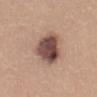The lesion was photographed on a routine skin check and not biopsied; there is no pathology result. Located on the abdomen. Imaged with white-light lighting. A female patient aged approximately 35. The recorded lesion diameter is about 5.5 mm. Automated tile analysis of the lesion measured an outline eccentricity of about 0.75 (0 = round, 1 = elongated). It also reported an average lesion color of about L≈48 a*≈19 b*≈23 (CIELAB) and about 17 CIELAB-L* units darker than the surrounding skin. The analysis additionally found an automated nevus-likeness rating near 60 out of 100 and a detector confidence of about 100 out of 100 that the crop contains a lesion. A 15 mm close-up extracted from a 3D total-body photography capture.